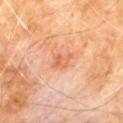Part of a total-body skin-imaging series; this lesion was reviewed on a skin check and was not flagged for biopsy.
The subject is a male aged approximately 60.
Approximately 2.5 mm at its widest.
The tile uses cross-polarized illumination.
The total-body-photography lesion software estimated a footprint of about 3.5 mm² and two-axis asymmetry of about 0.3. And it measured a mean CIELAB color near L≈62 a*≈27 b*≈37, a lesion–skin lightness drop of about 8, and a normalized lesion–skin contrast near 5.5. The software also gave a nevus-likeness score of about 0/100 and a detector confidence of about 100 out of 100 that the crop contains a lesion.
A 15 mm close-up extracted from a 3D total-body photography capture.
Located on the chest.